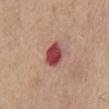workup: total-body-photography surveillance lesion; no biopsy | subject: male, approximately 75 years of age | site: the chest | image source: ~15 mm tile from a whole-body skin photo.